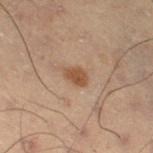The lesion was photographed on a routine skin check and not biopsied; there is no pathology result. The tile uses cross-polarized illumination. The lesion's longest dimension is about 3 mm. A male subject, approximately 55 years of age. From the right thigh. Automated image analysis of the tile measured an automated nevus-likeness rating near 80 out of 100 and a lesion-detection confidence of about 100/100. Cropped from a whole-body photographic skin survey; the tile spans about 15 mm.